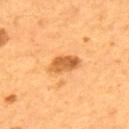Part of a total-body skin-imaging series; this lesion was reviewed on a skin check and was not flagged for biopsy. The patient is a male aged around 55. Captured under cross-polarized illumination. The recorded lesion diameter is about 4 mm. The lesion is on the upper back. A lesion tile, about 15 mm wide, cut from a 3D total-body photograph. Automated tile analysis of the lesion measured a lesion area of about 7 mm², an outline eccentricity of about 0.85 (0 = round, 1 = elongated), and a symmetry-axis asymmetry near 0.2. And it measured a border-irregularity rating of about 2/10 and a peripheral color-asymmetry measure near 1.5. The analysis additionally found a classifier nevus-likeness of about 60/100.